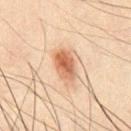<record>
<site>chest</site>
<patient>
  <sex>male</sex>
  <age_approx>35</age_approx>
</patient>
<automated_metrics>
  <area_mm2_approx>9.0</area_mm2_approx>
  <eccentricity>0.8</eccentricity>
  <vs_skin_darker_L>12.0</vs_skin_darker_L>
  <vs_skin_contrast_norm>8.5</vs_skin_contrast_norm>
  <nevus_likeness_0_100>100</nevus_likeness_0_100>
  <lesion_detection_confidence_0_100>100</lesion_detection_confidence_0_100>
</automated_metrics>
<lighting>cross-polarized</lighting>
<image>
  <source>total-body photography crop</source>
  <field_of_view_mm>15</field_of_view_mm>
</image>
<lesion_size>
  <long_diameter_mm_approx>4.0</long_diameter_mm_approx>
</lesion_size>
</record>